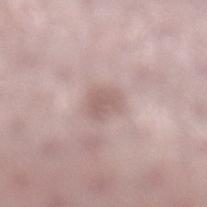<case>
<biopsy_status>not biopsied; imaged during a skin examination</biopsy_status>
<lighting>white-light</lighting>
<image>
  <source>total-body photography crop</source>
  <field_of_view_mm>15</field_of_view_mm>
</image>
<lesion_size>
  <long_diameter_mm_approx>3.0</long_diameter_mm_approx>
</lesion_size>
<patient>
  <sex>female</sex>
  <age_approx>45</age_approx>
</patient>
<automated_metrics>
  <area_mm2_approx>5.5</area_mm2_approx>
  <eccentricity>0.55</eccentricity>
  <cielab_L>60</cielab_L>
  <cielab_a>17</cielab_a>
  <cielab_b>20</cielab_b>
  <vs_skin_contrast_norm>5.5</vs_skin_contrast_norm>
</automated_metrics>
<site>left lower leg</site>
</case>